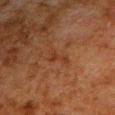Imaged during a routine full-body skin examination; the lesion was not biopsied and no histopathology is available. Located on the right upper arm. The tile uses cross-polarized illumination. Cropped from a total-body skin-imaging series; the visible field is about 15 mm. A male subject, in their 80s.